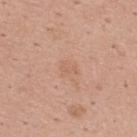No biopsy was performed on this lesion — it was imaged during a full skin examination and was not determined to be concerning.
On the upper back.
Approximately 2 mm at its widest.
A 15 mm crop from a total-body photograph taken for skin-cancer surveillance.
Automated tile analysis of the lesion measured an average lesion color of about L≈61 a*≈22 b*≈31 (CIELAB), about 5 CIELAB-L* units darker than the surrounding skin, and a lesion-to-skin contrast of about 4 (normalized; higher = more distinct). And it measured a border-irregularity index near 3.5/10 and peripheral color asymmetry of about 0.5. It also reported a detector confidence of about 100 out of 100 that the crop contains a lesion.
A male subject about 25 years old.